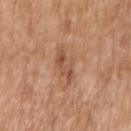No biopsy was performed on this lesion — it was imaged during a full skin examination and was not determined to be concerning.
A male subject, roughly 65 years of age.
Imaged with white-light lighting.
The lesion is on the right upper arm.
A roughly 15 mm field-of-view crop from a total-body skin photograph.